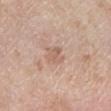Imaged with white-light lighting.
This image is a 15 mm lesion crop taken from a total-body photograph.
Longest diameter approximately 2.5 mm.
The total-body-photography lesion software estimated a footprint of about 3 mm², a shape eccentricity near 0.7, and two-axis asymmetry of about 0.4. The software also gave a mean CIELAB color near L≈60 a*≈19 b*≈28, roughly 7 lightness units darker than nearby skin, and a normalized border contrast of about 5. The software also gave border irregularity of about 4 on a 0–10 scale, internal color variation of about 1 on a 0–10 scale, and a peripheral color-asymmetry measure near 0.5.
From the leg.
A female subject, aged 68–72.